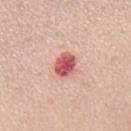Findings:
- follow-up · no biopsy performed (imaged during a skin exam)
- acquisition · total-body-photography crop, ~15 mm field of view
- subject · female, in their mid- to late 50s
- lesion diameter · about 3.5 mm
- tile lighting · white-light illumination
- location · the mid back